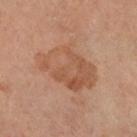No biopsy was performed on this lesion — it was imaged during a full skin examination and was not determined to be concerning.
A male patient aged around 65.
Automated tile analysis of the lesion measured a footprint of about 23 mm² and two-axis asymmetry of about 0.25. The software also gave an average lesion color of about L≈51 a*≈21 b*≈31 (CIELAB), roughly 7 lightness units darker than nearby skin, and a lesion-to-skin contrast of about 6 (normalized; higher = more distinct).
Captured under cross-polarized illumination.
On the left forearm.
Cropped from a whole-body photographic skin survey; the tile spans about 15 mm.